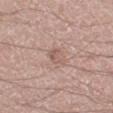Captured during whole-body skin photography for melanoma surveillance; the lesion was not biopsied. Located on the right lower leg. A male subject, aged approximately 50. A region of skin cropped from a whole-body photographic capture, roughly 15 mm wide.